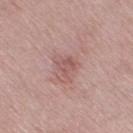Q: Was this lesion biopsied?
A: no biopsy performed (imaged during a skin exam)
Q: What kind of image is this?
A: 15 mm crop, total-body photography
Q: Who is the patient?
A: female, roughly 40 years of age
Q: Illumination type?
A: white-light illumination
Q: How large is the lesion?
A: ≈3 mm
Q: Lesion location?
A: the right thigh